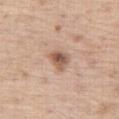  biopsy_status: not biopsied; imaged during a skin examination
  lighting: white-light
  site: right thigh
  image:
    source: total-body photography crop
    field_of_view_mm: 15
  patient:
    sex: male
    age_approx: 70